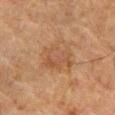Part of a total-body skin-imaging series; this lesion was reviewed on a skin check and was not flagged for biopsy.
Located on the abdomen.
The recorded lesion diameter is about 4.5 mm.
A roughly 15 mm field-of-view crop from a total-body skin photograph.
A male subject aged 78 to 82.
Captured under cross-polarized illumination.